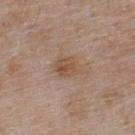The lesion was tiled from a total-body skin photograph and was not biopsied. A male patient, aged 53 to 57. Automated tile analysis of the lesion measured a nevus-likeness score of about 10/100. Longest diameter approximately 4 mm. The lesion is located on the upper back. Cropped from a total-body skin-imaging series; the visible field is about 15 mm. This is a white-light tile.